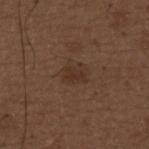follow-up = catalogued during a skin exam; not biopsied
subject = male, in their 50s
image source = ~15 mm crop, total-body skin-cancer survey
body site = the upper back
TBP lesion metrics = roughly 6 lightness units darker than nearby skin and a normalized border contrast of about 6.5; a border-irregularity index near 3.5/10, a within-lesion color-variation index near 1/10, and a peripheral color-asymmetry measure near 0.5; a detector confidence of about 100 out of 100 that the crop contains a lesion